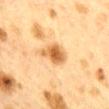<record>
  <biopsy_status>not biopsied; imaged during a skin examination</biopsy_status>
  <patient>
    <sex>female</sex>
    <age_approx>40</age_approx>
  </patient>
  <lighting>cross-polarized</lighting>
  <image>
    <source>total-body photography crop</source>
    <field_of_view_mm>15</field_of_view_mm>
  </image>
  <site>mid back</site>
  <automated_metrics>
    <area_mm2_approx>7.0</area_mm2_approx>
    <eccentricity>0.65</eccentricity>
    <shape_asymmetry>0.35</shape_asymmetry>
    <cielab_L>54</cielab_L>
    <cielab_a>20</cielab_a>
    <cielab_b>39</cielab_b>
    <vs_skin_darker_L>13.0</vs_skin_darker_L>
    <vs_skin_contrast_norm>9.5</vs_skin_contrast_norm>
    <nevus_likeness_0_100>85</nevus_likeness_0_100>
    <lesion_detection_confidence_0_100>100</lesion_detection_confidence_0_100>
  </automated_metrics>
  <lesion_size>
    <long_diameter_mm_approx>3.5</long_diameter_mm_approx>
  </lesion_size>
</record>